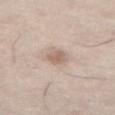Impression:
Part of a total-body skin-imaging series; this lesion was reviewed on a skin check and was not flagged for biopsy.
Background:
From the right thigh. A 15 mm crop from a total-body photograph taken for skin-cancer surveillance. A male subject, aged 73–77. Automated image analysis of the tile measured a peripheral color-asymmetry measure near 0.5. The software also gave an automated nevus-likeness rating near 75 out of 100. This is a white-light tile.